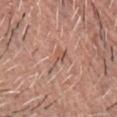{
  "biopsy_status": "not biopsied; imaged during a skin examination",
  "patient": {
    "sex": "male",
    "age_approx": 60
  },
  "image": {
    "source": "total-body photography crop",
    "field_of_view_mm": 15
  },
  "site": "chest"
}